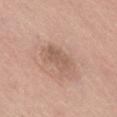This lesion was catalogued during total-body skin photography and was not selected for biopsy.
The patient is a male aged around 75.
The tile uses white-light illumination.
On the right thigh.
Cropped from a total-body skin-imaging series; the visible field is about 15 mm.
Approximately 3.5 mm at its widest.